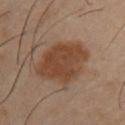workup = imaged on a skin check; not biopsied
anatomic site = the front of the torso
lighting = cross-polarized illumination
patient = male, approximately 40 years of age
TBP lesion metrics = an area of roughly 23 mm² and two-axis asymmetry of about 0.15; an average lesion color of about L≈42 a*≈19 b*≈30 (CIELAB), a lesion–skin lightness drop of about 10, and a normalized lesion–skin contrast near 9; a border-irregularity index near 2/10, internal color variation of about 3 on a 0–10 scale, and peripheral color asymmetry of about 1
diameter = ≈6.5 mm
image source = ~15 mm crop, total-body skin-cancer survey